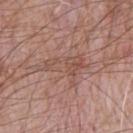<case>
<patient>
  <sex>male</sex>
  <age_approx>65</age_approx>
</patient>
<site>chest</site>
<lighting>white-light</lighting>
<lesion_size>
  <long_diameter_mm_approx>5.5</long_diameter_mm_approx>
</lesion_size>
<image>
  <source>total-body photography crop</source>
  <field_of_view_mm>15</field_of_view_mm>
</image>
</case>